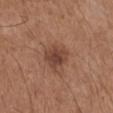Captured during whole-body skin photography for melanoma surveillance; the lesion was not biopsied. Imaged with white-light lighting. A region of skin cropped from a whole-body photographic capture, roughly 15 mm wide. The subject is a male approximately 75 years of age. The lesion-visualizer software estimated an outline eccentricity of about 0.6 (0 = round, 1 = elongated) and a symmetry-axis asymmetry near 0.25. It also reported a border-irregularity index near 2.5/10, internal color variation of about 4 on a 0–10 scale, and a peripheral color-asymmetry measure near 1. The analysis additionally found a detector confidence of about 100 out of 100 that the crop contains a lesion. Approximately 3.5 mm at its widest. The lesion is located on the right lower leg.